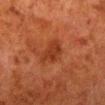* follow-up — catalogued during a skin exam; not biopsied
* patient — male, aged 78 to 82
* location — the left lower leg
* lesion diameter — ≈3.5 mm
* image source — 15 mm crop, total-body photography
* illumination — cross-polarized illumination